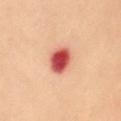Clinical impression:
Recorded during total-body skin imaging; not selected for excision or biopsy.
Clinical summary:
Cropped from a total-body skin-imaging series; the visible field is about 15 mm. An algorithmic analysis of the crop reported a footprint of about 8 mm². A female patient, aged around 60. This is a cross-polarized tile. The lesion is on the abdomen. The recorded lesion diameter is about 3.5 mm.